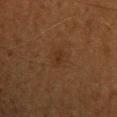Q: Is there a histopathology result?
A: total-body-photography surveillance lesion; no biopsy
Q: Patient demographics?
A: male, aged approximately 65
Q: Automated lesion metrics?
A: a mean CIELAB color near L≈25 a*≈16 b*≈25 and a normalized border contrast of about 5; a border-irregularity index near 2.5/10, a color-variation rating of about 1.5/10, and a peripheral color-asymmetry measure near 0.5
Q: What lighting was used for the tile?
A: cross-polarized
Q: What is the imaging modality?
A: ~15 mm tile from a whole-body skin photo
Q: Lesion size?
A: about 2.5 mm
Q: Lesion location?
A: the head or neck